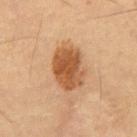Findings:
– notes — catalogued during a skin exam; not biopsied
– image — 15 mm crop, total-body photography
– location — the abdomen
– tile lighting — cross-polarized illumination
– size — about 5 mm
– subject — male, about 55 years old